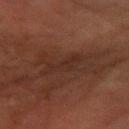notes — catalogued during a skin exam; not biopsied | lesion size — ~4.5 mm (longest diameter) | image source — total-body-photography crop, ~15 mm field of view | site — the left forearm | subject — male, about 70 years old | lighting — cross-polarized.